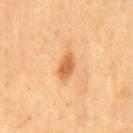| key | value |
|---|---|
| follow-up | imaged on a skin check; not biopsied |
| body site | the mid back |
| illumination | cross-polarized |
| image-analysis metrics | an average lesion color of about L≈64 a*≈25 b*≈46 (CIELAB); a color-variation rating of about 2/10; an automated nevus-likeness rating near 80 out of 100 |
| acquisition | ~15 mm crop, total-body skin-cancer survey |
| subject | male, roughly 50 years of age |
| lesion size | ≈3 mm |